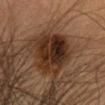follow-up: total-body-photography surveillance lesion; no biopsy